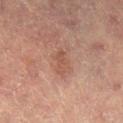<case>
<biopsy_status>not biopsied; imaged during a skin examination</biopsy_status>
<site>right lower leg</site>
<lighting>cross-polarized</lighting>
<image>
  <source>total-body photography crop</source>
  <field_of_view_mm>15</field_of_view_mm>
</image>
<patient>
  <sex>female</sex>
  <age_approx>70</age_approx>
</patient>
<automated_metrics>
  <vs_skin_contrast_norm>5.0</vs_skin_contrast_norm>
</automated_metrics>
<lesion_size>
  <long_diameter_mm_approx>3.0</long_diameter_mm_approx>
</lesion_size>
</case>